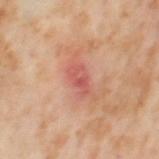Impression: Recorded during total-body skin imaging; not selected for excision or biopsy. Image and clinical context: From the left thigh. The lesion's longest dimension is about 3.5 mm. Cropped from a total-body skin-imaging series; the visible field is about 15 mm. A female patient, aged around 55.